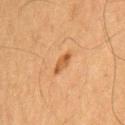This lesion was catalogued during total-body skin photography and was not selected for biopsy. A region of skin cropped from a whole-body photographic capture, roughly 15 mm wide. Measured at roughly 3 mm in maximum diameter. A male subject, roughly 65 years of age. This is a cross-polarized tile. On the front of the torso. An algorithmic analysis of the crop reported a lesion area of about 3 mm², a shape eccentricity near 0.95, and a symmetry-axis asymmetry near 0.3. The software also gave an average lesion color of about L≈47 a*≈22 b*≈37 (CIELAB) and about 9 CIELAB-L* units darker than the surrounding skin. It also reported a border-irregularity index near 3.5/10 and internal color variation of about 0.5 on a 0–10 scale. And it measured an automated nevus-likeness rating near 85 out of 100 and a lesion-detection confidence of about 100/100.